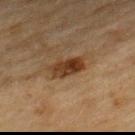This lesion was catalogued during total-body skin photography and was not selected for biopsy. The patient is a male approximately 70 years of age. This image is a 15 mm lesion crop taken from a total-body photograph. Captured under cross-polarized illumination. An algorithmic analysis of the crop reported a lesion area of about 7.5 mm², a shape eccentricity near 0.85, and a shape-asymmetry score of about 0.2 (0 = symmetric). The analysis additionally found border irregularity of about 2.5 on a 0–10 scale, internal color variation of about 5.5 on a 0–10 scale, and a peripheral color-asymmetry measure near 2. It also reported a detector confidence of about 100 out of 100 that the crop contains a lesion. The recorded lesion diameter is about 4.5 mm. Located on the back.